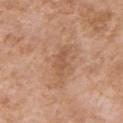Findings:
– follow-up — total-body-photography surveillance lesion; no biopsy
– body site — the arm
– automated metrics — a footprint of about 4 mm², an eccentricity of roughly 0.95, and two-axis asymmetry of about 0.35
– acquisition — ~15 mm tile from a whole-body skin photo
– lesion diameter — about 4 mm
– patient — female, aged around 75